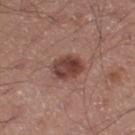Clinical impression:
Captured during whole-body skin photography for melanoma surveillance; the lesion was not biopsied.
Context:
A 15 mm crop from a total-body photograph taken for skin-cancer surveillance. The recorded lesion diameter is about 3.5 mm. Located on the left thigh. Imaged with white-light lighting. The patient is a male about 55 years old. Automated image analysis of the tile measured a lesion area of about 8.5 mm², a shape eccentricity near 0.7, and two-axis asymmetry of about 0.15. It also reported a mean CIELAB color near L≈42 a*≈22 b*≈24, a lesion–skin lightness drop of about 12, and a normalized border contrast of about 9.5. It also reported border irregularity of about 1.5 on a 0–10 scale, a color-variation rating of about 5/10, and a peripheral color-asymmetry measure near 2. The software also gave lesion-presence confidence of about 100/100.